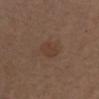notes: total-body-photography surveillance lesion; no biopsy
subject: female, approximately 40 years of age
anatomic site: the chest
lesion size: ~3 mm (longest diameter)
automated metrics: a lesion area of about 5.5 mm², an outline eccentricity of about 0.7 (0 = round, 1 = elongated), and two-axis asymmetry of about 0.25; a normalized border contrast of about 5.5; a border-irregularity rating of about 2.5/10, a color-variation rating of about 1.5/10, and radial color variation of about 0.5
image: 15 mm crop, total-body photography
lighting: white-light illumination